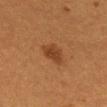* subject — female, aged 53–57
* diameter — ~3 mm (longest diameter)
* location — the mid back
* imaging modality — ~15 mm tile from a whole-body skin photo
* illumination — cross-polarized illumination